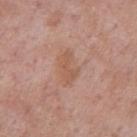Impression:
Part of a total-body skin-imaging series; this lesion was reviewed on a skin check and was not flagged for biopsy.
Background:
Located on the arm. The patient is a male in their mid- to late 50s. A 15 mm close-up tile from a total-body photography series done for melanoma screening.